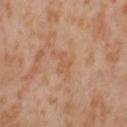<lesion>
<biopsy_status>not biopsied; imaged during a skin examination</biopsy_status>
<site>left thigh</site>
<lighting>cross-polarized</lighting>
<lesion_size>
  <long_diameter_mm_approx>4.0</long_diameter_mm_approx>
</lesion_size>
<image>
  <source>total-body photography crop</source>
  <field_of_view_mm>15</field_of_view_mm>
</image>
<patient>
  <sex>female</sex>
  <age_approx>55</age_approx>
</patient>
</lesion>